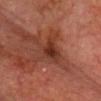Case summary:
- notes · imaged on a skin check; not biopsied
- anatomic site · the front of the torso
- automated lesion analysis · a border-irregularity index near 4/10, a within-lesion color-variation index near 3.5/10, and radial color variation of about 1; an automated nevus-likeness rating near 10 out of 100 and a detector confidence of about 90 out of 100 that the crop contains a lesion
- tile lighting · cross-polarized
- diameter · about 4.5 mm
- patient · male, aged 73 to 77
- image · ~15 mm crop, total-body skin-cancer survey